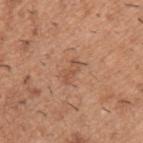Q: Was a biopsy performed?
A: catalogued during a skin exam; not biopsied
Q: How large is the lesion?
A: ≈3 mm
Q: Illumination type?
A: white-light
Q: Patient demographics?
A: male, roughly 40 years of age
Q: Lesion location?
A: the back
Q: How was this image acquired?
A: ~15 mm crop, total-body skin-cancer survey